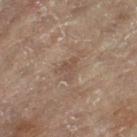  biopsy_status: not biopsied; imaged during a skin examination